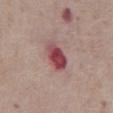Notes:
– notes — catalogued during a skin exam; not biopsied
– lesion diameter — ~4 mm (longest diameter)
– lighting — white-light
– image — 15 mm crop, total-body photography
– patient — male, in their mid-70s
– anatomic site — the front of the torso
– image-analysis metrics — a border-irregularity index near 1.5/10 and a peripheral color-asymmetry measure near 2.5